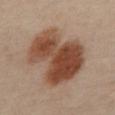Clinical impression:
Imaged during a routine full-body skin examination; the lesion was not biopsied and no histopathology is available.
Clinical summary:
Cropped from a total-body skin-imaging series; the visible field is about 15 mm. From the mid back. A male patient about 65 years old. The tile uses cross-polarized illumination.